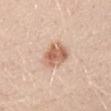Clinical impression: Imaged during a routine full-body skin examination; the lesion was not biopsied and no histopathology is available. Clinical summary: The lesion-visualizer software estimated an area of roughly 8.5 mm², an outline eccentricity of about 0.4 (0 = round, 1 = elongated), and two-axis asymmetry of about 0.15. The software also gave a mean CIELAB color near L≈63 a*≈22 b*≈31 and about 13 CIELAB-L* units darker than the surrounding skin. A 15 mm close-up extracted from a 3D total-body photography capture. A female patient, aged 38 to 42. The lesion is on the mid back. Measured at roughly 3.5 mm in maximum diameter.